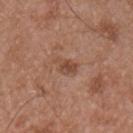biopsy status: catalogued during a skin exam; not biopsied
site: the chest
tile lighting: white-light
automated metrics: a lesion area of about 4.5 mm², an outline eccentricity of about 0.85 (0 = round, 1 = elongated), and a symmetry-axis asymmetry near 0.3; border irregularity of about 3.5 on a 0–10 scale and a within-lesion color-variation index near 1.5/10; an automated nevus-likeness rating near 5 out of 100
patient: male, about 55 years old
lesion diameter: about 3 mm
image: ~15 mm tile from a whole-body skin photo